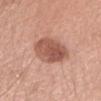Clinical summary: The patient is a female approximately 40 years of age. A close-up tile cropped from a whole-body skin photograph, about 15 mm across. From the head or neck.